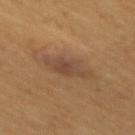This lesion was catalogued during total-body skin photography and was not selected for biopsy.
The lesion is located on the mid back.
The patient is a male about 50 years old.
A region of skin cropped from a whole-body photographic capture, roughly 15 mm wide.